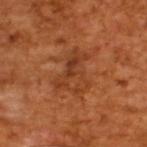Assessment: Imaged during a routine full-body skin examination; the lesion was not biopsied and no histopathology is available. Clinical summary: The tile uses cross-polarized illumination. The lesion's longest dimension is about 5 mm. The patient is a male aged 63 to 67. A 15 mm close-up tile from a total-body photography series done for melanoma screening. Automated image analysis of the tile measured a lesion color around L≈39 a*≈26 b*≈36 in CIELAB, roughly 7 lightness units darker than nearby skin, and a normalized lesion–skin contrast near 6.